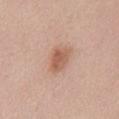Recorded during total-body skin imaging; not selected for excision or biopsy.
From the back.
A 15 mm close-up extracted from a 3D total-body photography capture.
A female subject, aged around 50.